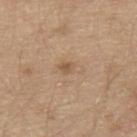• biopsy status — no biopsy performed (imaged during a skin exam)
• body site — the mid back
• image-analysis metrics — an area of roughly 4.5 mm², an eccentricity of roughly 0.85, and two-axis asymmetry of about 0.35; a lesion color around L≈57 a*≈16 b*≈32 in CIELAB, a lesion–skin lightness drop of about 7, and a normalized lesion–skin contrast near 5; a border-irregularity index near 3.5/10 and a within-lesion color-variation index near 4.5/10; a nevus-likeness score of about 5/100 and a lesion-detection confidence of about 100/100
• subject — male, aged around 70
• lesion size — ≈3.5 mm
• image — 15 mm crop, total-body photography